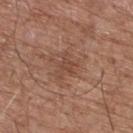follow-up = catalogued during a skin exam; not biopsied
image source = ~15 mm crop, total-body skin-cancer survey
anatomic site = the upper back
subject = male, aged around 75